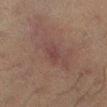| field | value |
|---|---|
| follow-up | total-body-photography surveillance lesion; no biopsy |
| site | the leg |
| patient | male, in their 50s |
| automated lesion analysis | a footprint of about 3.5 mm², an outline eccentricity of about 0.8 (0 = round, 1 = elongated), and a symmetry-axis asymmetry near 0.3; a mean CIELAB color near L≈31 a*≈16 b*≈17 and about 5 CIELAB-L* units darker than the surrounding skin; a border-irregularity rating of about 2.5/10 and radial color variation of about 0.5 |
| imaging modality | total-body-photography crop, ~15 mm field of view |